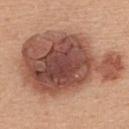The lesion was tiled from a total-body skin photograph and was not biopsied. Cropped from a total-body skin-imaging series; the visible field is about 15 mm. The lesion is located on the back. The tile uses white-light illumination. The subject is a female roughly 45 years of age. Approximately 12.5 mm at its widest.